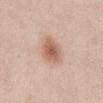Captured during whole-body skin photography for melanoma surveillance; the lesion was not biopsied.
The lesion is located on the abdomen.
A 15 mm crop from a total-body photograph taken for skin-cancer surveillance.
The lesion-visualizer software estimated a lesion area of about 8.5 mm² and a shape-asymmetry score of about 0.2 (0 = symmetric). The software also gave a lesion color around L≈61 a*≈21 b*≈29 in CIELAB and roughly 12 lightness units darker than nearby skin. The analysis additionally found border irregularity of about 2 on a 0–10 scale, a within-lesion color-variation index near 3.5/10, and peripheral color asymmetry of about 1. The analysis additionally found an automated nevus-likeness rating near 95 out of 100.
A female subject, about 40 years old.
Captured under white-light illumination.